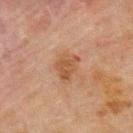biopsy status=imaged on a skin check; not biopsied | site=the upper back | imaging modality=~15 mm tile from a whole-body skin photo | subject=male, about 70 years old | lighting=cross-polarized illumination | automated metrics=a border-irregularity rating of about 3/10, a color-variation rating of about 2/10, and peripheral color asymmetry of about 0.5; a classifier nevus-likeness of about 0/100 and lesion-presence confidence of about 100/100 | lesion size=≈3 mm.